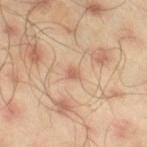site: the right thigh
patient: male, aged around 45
image source: total-body-photography crop, ~15 mm field of view